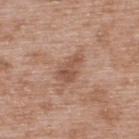The lesion is on the upper back. A male subject in their 50s. A close-up tile cropped from a whole-body skin photograph, about 15 mm across.